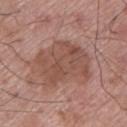Findings:
• notes: no biopsy performed (imaged during a skin exam)
• subject: male, roughly 70 years of age
• size: about 6.5 mm
• body site: the leg
• image: ~15 mm tile from a whole-body skin photo